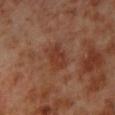Clinical impression:
This lesion was catalogued during total-body skin photography and was not selected for biopsy.
Clinical summary:
The tile uses cross-polarized illumination. The lesion is located on the leg. A male subject, aged 68 to 72. This image is a 15 mm lesion crop taken from a total-body photograph. The lesion's longest dimension is about 3.5 mm.